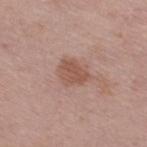No biopsy was performed on this lesion — it was imaged during a full skin examination and was not determined to be concerning. A 15 mm close-up extracted from a 3D total-body photography capture. An algorithmic analysis of the crop reported a shape eccentricity near 0.6 and two-axis asymmetry of about 0.25. The subject is a female aged approximately 55. On the right thigh.